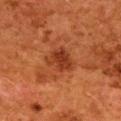Q: Was a biopsy performed?
A: imaged on a skin check; not biopsied
Q: Automated lesion metrics?
A: an area of roughly 8 mm² and an eccentricity of roughly 0.7; a lesion color around L≈32 a*≈26 b*≈32 in CIELAB, about 8 CIELAB-L* units darker than the surrounding skin, and a lesion-to-skin contrast of about 7.5 (normalized; higher = more distinct); border irregularity of about 3 on a 0–10 scale and radial color variation of about 1.5
Q: Lesion size?
A: about 4 mm
Q: What lighting was used for the tile?
A: cross-polarized illumination
Q: What is the anatomic site?
A: the upper back
Q: What kind of image is this?
A: ~15 mm tile from a whole-body skin photo
Q: Who is the patient?
A: female, aged 48 to 52